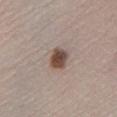Case summary:
• follow-up — catalogued during a skin exam; not biopsied
• size — about 3 mm
• automated lesion analysis — a mean CIELAB color near L≈46 a*≈17 b*≈23, roughly 16 lightness units darker than nearby skin, and a normalized border contrast of about 11.5; lesion-presence confidence of about 100/100
• acquisition — ~15 mm crop, total-body skin-cancer survey
• patient — male, aged 63 to 67
• illumination — white-light
• body site — the leg